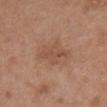Recorded during total-body skin imaging; not selected for excision or biopsy.
On the chest.
Automated image analysis of the tile measured an area of roughly 8 mm², an outline eccentricity of about 0.8 (0 = round, 1 = elongated), and a symmetry-axis asymmetry near 0.3. The analysis additionally found a normalized lesion–skin contrast near 5.5.
A roughly 15 mm field-of-view crop from a total-body skin photograph.
Imaged with white-light lighting.
The subject is a female in their 40s.